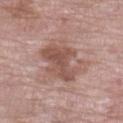Clinical impression: Imaged during a routine full-body skin examination; the lesion was not biopsied and no histopathology is available. Image and clinical context: The lesion is located on the left lower leg. A 15 mm close-up extracted from a 3D total-body photography capture. The tile uses white-light illumination. A female patient, approximately 70 years of age.